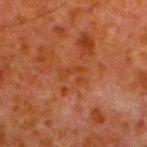Q: Is there a histopathology result?
A: catalogued during a skin exam; not biopsied